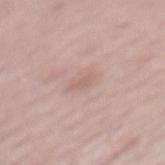biopsy status: no biopsy performed (imaged during a skin exam) | location: the mid back | image: total-body-photography crop, ~15 mm field of view | patient: female, aged approximately 50 | size: ~2.5 mm (longest diameter).